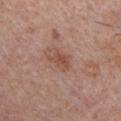– follow-up — imaged on a skin check; not biopsied
– subject — male, aged 28–32
– image source — ~15 mm crop, total-body skin-cancer survey
– lesion diameter — about 3 mm
– image-analysis metrics — a lesion color around L≈50 a*≈22 b*≈28 in CIELAB, roughly 8 lightness units darker than nearby skin, and a normalized lesion–skin contrast near 6.5; a classifier nevus-likeness of about 15/100 and lesion-presence confidence of about 100/100
– lighting — white-light
– body site — the chest